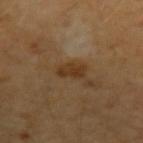The subject is a male about 45 years old. This image is a 15 mm lesion crop taken from a total-body photograph. Captured under cross-polarized illumination. Located on the arm.